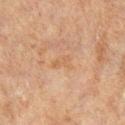About 2.5 mm across. Automated image analysis of the tile measured an area of roughly 3.5 mm², an eccentricity of roughly 0.9, and a symmetry-axis asymmetry near 0.3. It also reported a border-irregularity rating of about 3/10, a color-variation rating of about 0.5/10, and peripheral color asymmetry of about 0. And it measured a classifier nevus-likeness of about 0/100. A male subject in their 70s. The lesion is on the right lower leg. The tile uses cross-polarized illumination. Cropped from a total-body skin-imaging series; the visible field is about 15 mm.